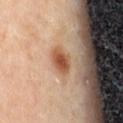patient — female, aged around 50
image source — 15 mm crop, total-body photography
size — ~3 mm (longest diameter)
tile lighting — cross-polarized
TBP lesion metrics — an area of roughly 6 mm², an outline eccentricity of about 0.7 (0 = round, 1 = elongated), and a shape-asymmetry score of about 0.15 (0 = symmetric); a mean CIELAB color near L≈52 a*≈22 b*≈34, roughly 12 lightness units darker than nearby skin, and a normalized border contrast of about 9; border irregularity of about 1.5 on a 0–10 scale, a within-lesion color-variation index near 3.5/10, and a peripheral color-asymmetry measure near 1
body site — the abdomen